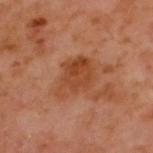The lesion was photographed on a routine skin check and not biopsied; there is no pathology result.
From the upper back.
A 15 mm close-up extracted from a 3D total-body photography capture.
Measured at roughly 4.5 mm in maximum diameter.
The tile uses cross-polarized illumination.
A male subject roughly 60 years of age.